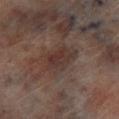Recorded during total-body skin imaging; not selected for excision or biopsy. Automated image analysis of the tile measured an automated nevus-likeness rating near 0 out of 100 and lesion-presence confidence of about 95/100. The tile uses cross-polarized illumination. The subject is a male roughly 65 years of age. Located on the left lower leg. The lesion's longest dimension is about 4.5 mm. This image is a 15 mm lesion crop taken from a total-body photograph.